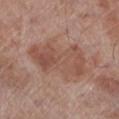Recorded during total-body skin imaging; not selected for excision or biopsy. The recorded lesion diameter is about 8 mm. The lesion-visualizer software estimated a lesion area of about 25 mm², an eccentricity of roughly 0.85, and a symmetry-axis asymmetry near 0.2. It also reported about 8 CIELAB-L* units darker than the surrounding skin. And it measured border irregularity of about 3.5 on a 0–10 scale, internal color variation of about 4.5 on a 0–10 scale, and radial color variation of about 1.5. The analysis additionally found a classifier nevus-likeness of about 0/100 and lesion-presence confidence of about 100/100. The subject is a male roughly 70 years of age. The tile uses white-light illumination. A 15 mm crop from a total-body photograph taken for skin-cancer surveillance. Located on the leg.